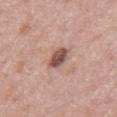A roughly 15 mm field-of-view crop from a total-body skin photograph.
A male patient roughly 55 years of age.
Located on the mid back.